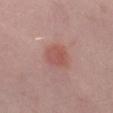Recorded during total-body skin imaging; not selected for excision or biopsy.
Automated image analysis of the tile measured a footprint of about 6.5 mm², a shape eccentricity near 0.55, and two-axis asymmetry of about 0.2.
A male subject, aged around 40.
Located on the left thigh.
Captured under white-light illumination.
A close-up tile cropped from a whole-body skin photograph, about 15 mm across.